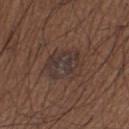Captured during whole-body skin photography for melanoma surveillance; the lesion was not biopsied. Longest diameter approximately 5 mm. The lesion-visualizer software estimated a lesion area of about 15 mm² and an outline eccentricity of about 0.65 (0 = round, 1 = elongated). And it measured an average lesion color of about L≈34 a*≈14 b*≈19 (CIELAB) and about 5 CIELAB-L* units darker than the surrounding skin. Imaged with white-light lighting. Cropped from a total-body skin-imaging series; the visible field is about 15 mm. From the left thigh. A male subject, aged 63 to 67.